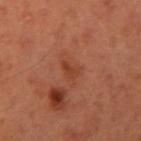This lesion was catalogued during total-body skin photography and was not selected for biopsy. Automated tile analysis of the lesion measured about 6 CIELAB-L* units darker than the surrounding skin and a normalized lesion–skin contrast near 6. A region of skin cropped from a whole-body photographic capture, roughly 15 mm wide. The lesion is located on the right upper arm. The subject is a male aged around 35. Approximately 2 mm at its widest.